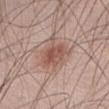The lesion was photographed on a routine skin check and not biopsied; there is no pathology result. Cropped from a total-body skin-imaging series; the visible field is about 15 mm. On the abdomen. Measured at roughly 4 mm in maximum diameter. Imaged with white-light lighting. The total-body-photography lesion software estimated a lesion area of about 12 mm², a shape eccentricity near 0.5, and a shape-asymmetry score of about 0.3 (0 = symmetric). And it measured an automated nevus-likeness rating near 90 out of 100 and a lesion-detection confidence of about 100/100. A male patient, roughly 60 years of age.